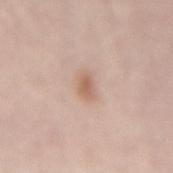The lesion was photographed on a routine skin check and not biopsied; there is no pathology result. Imaged with white-light lighting. A female subject aged around 65. About 2.5 mm across. An algorithmic analysis of the crop reported a shape eccentricity near 0.8 and a shape-asymmetry score of about 0.25 (0 = symmetric). And it measured a border-irregularity rating of about 2.5/10, a within-lesion color-variation index near 2/10, and radial color variation of about 0.5. A close-up tile cropped from a whole-body skin photograph, about 15 mm across. The lesion is located on the lower back.